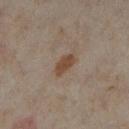Recorded during total-body skin imaging; not selected for excision or biopsy. The tile uses cross-polarized illumination. The lesion is on the leg. A roughly 15 mm field-of-view crop from a total-body skin photograph. Longest diameter approximately 3 mm. A female patient aged around 35.